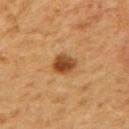<lesion>
  <biopsy_status>not biopsied; imaged during a skin examination</biopsy_status>
  <lesion_size>
    <long_diameter_mm_approx>3.0</long_diameter_mm_approx>
  </lesion_size>
  <patient>
    <sex>male</sex>
    <age_approx>60</age_approx>
  </patient>
  <automated_metrics>
    <area_mm2_approx>6.0</area_mm2_approx>
    <eccentricity>0.55</eccentricity>
    <shape_asymmetry>0.2</shape_asymmetry>
    <vs_skin_darker_L>14.0</vs_skin_darker_L>
    <vs_skin_contrast_norm>10.0</vs_skin_contrast_norm>
    <nevus_likeness_0_100>100</nevus_likeness_0_100>
    <lesion_detection_confidence_0_100>100</lesion_detection_confidence_0_100>
  </automated_metrics>
  <image>
    <source>total-body photography crop</source>
    <field_of_view_mm>15</field_of_view_mm>
  </image>
  <site>mid back</site>
  <lighting>cross-polarized</lighting>
</lesion>